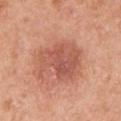The lesion was tiled from a total-body skin photograph and was not biopsied. From the right upper arm. Automated image analysis of the tile measured an average lesion color of about L≈56 a*≈27 b*≈30 (CIELAB) and a lesion–skin lightness drop of about 10. The analysis additionally found a border-irregularity rating of about 5.5/10, a within-lesion color-variation index near 4.5/10, and peripheral color asymmetry of about 1.5. A region of skin cropped from a whole-body photographic capture, roughly 15 mm wide. The patient is a female in their 40s. The lesion's longest dimension is about 6 mm. This is a white-light tile.